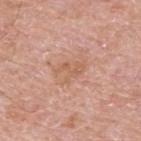Impression:
The lesion was tiled from a total-body skin photograph and was not biopsied.
Context:
A close-up tile cropped from a whole-body skin photograph, about 15 mm across. Approximately 3 mm at its widest. The lesion-visualizer software estimated an average lesion color of about L≈60 a*≈22 b*≈32 (CIELAB), roughly 7 lightness units darker than nearby skin, and a normalized border contrast of about 5.5. And it measured a classifier nevus-likeness of about 0/100. On the upper back. A male subject aged around 65. Captured under white-light illumination.